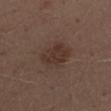Notes:
* image — 15 mm crop, total-body photography
* size — ~4 mm (longest diameter)
* body site — the left thigh
* TBP lesion metrics — a lesion color around L≈32 a*≈16 b*≈22 in CIELAB, roughly 7 lightness units darker than nearby skin, and a normalized lesion–skin contrast near 7; a border-irregularity index near 2.5/10 and peripheral color asymmetry of about 1
* tile lighting — white-light
* patient — male, roughly 70 years of age